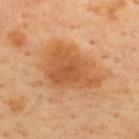This lesion was catalogued during total-body skin photography and was not selected for biopsy. Captured under cross-polarized illumination. On the upper back. A male patient, in their mid- to late 60s. This image is a 15 mm lesion crop taken from a total-body photograph. The lesion's longest dimension is about 6.5 mm.